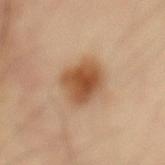notes: total-body-photography surveillance lesion; no biopsy
image source: ~15 mm crop, total-body skin-cancer survey
patient: male, aged 48–52
lesion size: ≈4.5 mm
anatomic site: the mid back